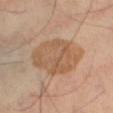tile lighting = cross-polarized | patient = female, roughly 60 years of age | site = the left thigh | image = total-body-photography crop, ~15 mm field of view.